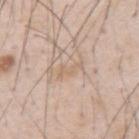The lesion was tiled from a total-body skin photograph and was not biopsied.
The subject is a male aged 53 to 57.
About 3 mm across.
Cropped from a whole-body photographic skin survey; the tile spans about 15 mm.
Captured under white-light illumination.